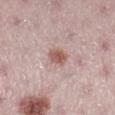Imaged during a routine full-body skin examination; the lesion was not biopsied and no histopathology is available.
The tile uses white-light illumination.
The recorded lesion diameter is about 3 mm.
Automated image analysis of the tile measured a border-irregularity rating of about 2/10 and a within-lesion color-variation index near 3/10. And it measured a nevus-likeness score of about 90/100.
A female subject, about 25 years old.
Located on the right lower leg.
A lesion tile, about 15 mm wide, cut from a 3D total-body photograph.